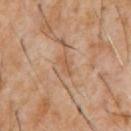Recorded during total-body skin imaging; not selected for excision or biopsy. This is a cross-polarized tile. Approximately 2.5 mm at its widest. A male subject aged 58–62. A region of skin cropped from a whole-body photographic capture, roughly 15 mm wide. The lesion is located on the chest.